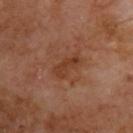This lesion was catalogued during total-body skin photography and was not selected for biopsy. Automated tile analysis of the lesion measured an area of roughly 5 mm², a shape eccentricity near 0.9, and a shape-asymmetry score of about 0.3 (0 = symmetric). And it measured an average lesion color of about L≈37 a*≈23 b*≈31 (CIELAB) and roughly 7 lightness units darker than nearby skin. It also reported an automated nevus-likeness rating near 0 out of 100 and lesion-presence confidence of about 100/100. A 15 mm close-up tile from a total-body photography series done for melanoma screening. A male patient aged 68 to 72. Approximately 3.5 mm at its widest. From the upper back. Captured under cross-polarized illumination.